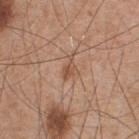follow-up: no biopsy performed (imaged during a skin exam)
size: ≈2.5 mm
illumination: white-light illumination
automated metrics: a shape eccentricity near 0.8 and a shape-asymmetry score of about 0.4 (0 = symmetric); an average lesion color of about L≈52 a*≈20 b*≈31 (CIELAB), roughly 9 lightness units darker than nearby skin, and a lesion-to-skin contrast of about 6.5 (normalized; higher = more distinct); a border-irregularity index near 4.5/10, a color-variation rating of about 2.5/10, and a peripheral color-asymmetry measure near 1; a nevus-likeness score of about 10/100
anatomic site: the chest
subject: male, roughly 55 years of age
imaging modality: ~15 mm crop, total-body skin-cancer survey